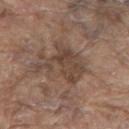workup = imaged on a skin check; not biopsied
lesion size = ~5.5 mm (longest diameter)
illumination = white-light
site = the upper back
TBP lesion metrics = an area of roughly 15 mm² and a shape eccentricity near 0.55; a mean CIELAB color near L≈43 a*≈15 b*≈25, roughly 8 lightness units darker than nearby skin, and a normalized lesion–skin contrast near 7; an automated nevus-likeness rating near 0 out of 100 and lesion-presence confidence of about 65/100
subject = male, aged 78 to 82
image source = total-body-photography crop, ~15 mm field of view